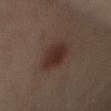{
  "biopsy_status": "not biopsied; imaged during a skin examination",
  "patient": {
    "sex": "female",
    "age_approx": 60
  },
  "image": {
    "source": "total-body photography crop",
    "field_of_view_mm": 15
  },
  "automated_metrics": {
    "area_mm2_approx": 10.0,
    "eccentricity": 0.75,
    "shape_asymmetry": 0.15,
    "border_irregularity_0_10": 2.0,
    "color_variation_0_10": 3.0,
    "peripheral_color_asymmetry": 1.0
  },
  "site": "mid back"
}